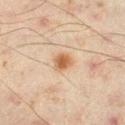A lesion tile, about 15 mm wide, cut from a 3D total-body photograph.
Automated image analysis of the tile measured a lesion color around L≈52 a*≈19 b*≈33 in CIELAB, a lesion–skin lightness drop of about 11, and a normalized lesion–skin contrast near 9. The software also gave a classifier nevus-likeness of about 100/100 and a lesion-detection confidence of about 100/100.
On the right lower leg.
This is a cross-polarized tile.
Longest diameter approximately 2.5 mm.
A male patient, in their mid- to late 40s.